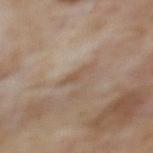follow-up = imaged on a skin check; not biopsied
image = ~15 mm crop, total-body skin-cancer survey
body site = the upper back
patient = female, aged 58 to 62